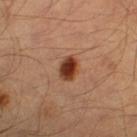Imaged during a routine full-body skin examination; the lesion was not biopsied and no histopathology is available. From the right lower leg. Cropped from a whole-body photographic skin survey; the tile spans about 15 mm. The lesion's longest dimension is about 3 mm. A male patient aged 38 to 42.